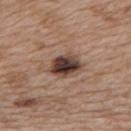Case summary:
• workup: no biopsy performed (imaged during a skin exam)
• imaging modality: ~15 mm tile from a whole-body skin photo
• lighting: white-light
• patient: female, aged 38 to 42
• location: the upper back
• automated lesion analysis: a mean CIELAB color near L≈40 a*≈17 b*≈23 and a lesion-to-skin contrast of about 13.5 (normalized; higher = more distinct); a border-irregularity rating of about 2/10, a within-lesion color-variation index near 8.5/10, and peripheral color asymmetry of about 2.5